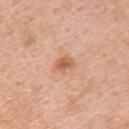notes: catalogued during a skin exam; not biopsied | patient: male, approximately 60 years of age | acquisition: ~15 mm tile from a whole-body skin photo | location: the upper back.